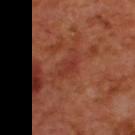Impression: No biopsy was performed on this lesion — it was imaged during a full skin examination and was not determined to be concerning. Clinical summary: A male subject roughly 50 years of age. From the upper back. About 2.5 mm across. Automated tile analysis of the lesion measured a mean CIELAB color near L≈36 a*≈30 b*≈30, roughly 5 lightness units darker than nearby skin, and a lesion-to-skin contrast of about 5 (normalized; higher = more distinct). The analysis additionally found border irregularity of about 3 on a 0–10 scale, a within-lesion color-variation index near 2.5/10, and peripheral color asymmetry of about 1. The software also gave a detector confidence of about 100 out of 100 that the crop contains a lesion. This image is a 15 mm lesion crop taken from a total-body photograph.